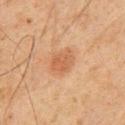workup = catalogued during a skin exam; not biopsied
location = the chest
image = total-body-photography crop, ~15 mm field of view
subject = male, aged 58 to 62